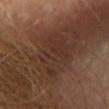Context: The lesion is on the chest. A female subject, aged approximately 65. A 15 mm crop from a total-body photograph taken for skin-cancer surveillance. The tile uses cross-polarized illumination. The lesion's longest dimension is about 6 mm.